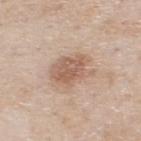workup=no biopsy performed (imaged during a skin exam)
illumination=white-light illumination
lesion size=~5 mm (longest diameter)
anatomic site=the upper back
acquisition=total-body-photography crop, ~15 mm field of view
patient=male, aged around 80
TBP lesion metrics=a footprint of about 13 mm² and a symmetry-axis asymmetry near 0.25; an average lesion color of about L≈60 a*≈18 b*≈28 (CIELAB), a lesion–skin lightness drop of about 10, and a normalized lesion–skin contrast near 7; a border-irregularity rating of about 3/10, a within-lesion color-variation index near 3.5/10, and a peripheral color-asymmetry measure near 1; an automated nevus-likeness rating near 35 out of 100 and lesion-presence confidence of about 100/100